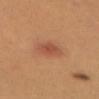| feature | finding |
|---|---|
| follow-up | total-body-photography surveillance lesion; no biopsy |
| body site | the chest |
| acquisition | 15 mm crop, total-body photography |
| lighting | white-light illumination |
| subject | female, aged 23–27 |